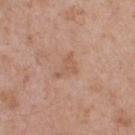The lesion was photographed on a routine skin check and not biopsied; there is no pathology result. A close-up tile cropped from a whole-body skin photograph, about 15 mm across. From the upper back. About 3 mm across. The tile uses white-light illumination. Automated image analysis of the tile measured a lesion area of about 4 mm², an eccentricity of roughly 0.75, and two-axis asymmetry of about 0.6. The analysis additionally found lesion-presence confidence of about 100/100. The subject is a male roughly 55 years of age.